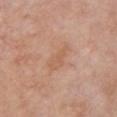workup: total-body-photography surveillance lesion; no biopsy
diameter: ≈4 mm
illumination: white-light
anatomic site: the chest
image: total-body-photography crop, ~15 mm field of view
patient: female, in their 60s